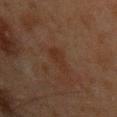Located on the left upper arm.
A male subject, aged around 45.
This image is a 15 mm lesion crop taken from a total-body photograph.